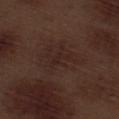notes = total-body-photography surveillance lesion; no biopsy
site = the right thigh
image = 15 mm crop, total-body photography
size = about 2.5 mm
lighting = white-light
automated lesion analysis = a mean CIELAB color near L≈22 a*≈18 b*≈19 and roughly 4 lightness units darker than nearby skin; an automated nevus-likeness rating near 0 out of 100 and lesion-presence confidence of about 95/100
subject = male, in their 70s